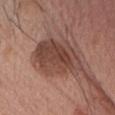Case summary:
- biopsy status — total-body-photography surveillance lesion; no biopsy
- illumination — white-light illumination
- subject — female, aged 58 to 62
- image source — total-body-photography crop, ~15 mm field of view
- location — the head or neck
- lesion size — about 6.5 mm